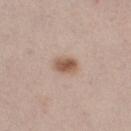The lesion was photographed on a routine skin check and not biopsied; there is no pathology result. The total-body-photography lesion software estimated a shape eccentricity near 0.75 and a shape-asymmetry score of about 0.2 (0 = symmetric). And it measured a lesion color around L≈56 a*≈18 b*≈28 in CIELAB, roughly 13 lightness units darker than nearby skin, and a normalized lesion–skin contrast near 9. And it measured a border-irregularity rating of about 1.5/10 and peripheral color asymmetry of about 1. And it measured a nevus-likeness score of about 100/100 and a lesion-detection confidence of about 100/100. Measured at roughly 3 mm in maximum diameter. The subject is a female about 40 years old. Located on the right thigh. The tile uses white-light illumination. A 15 mm close-up extracted from a 3D total-body photography capture.